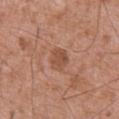notes: no biopsy performed (imaged during a skin exam); anatomic site: the abdomen; patient: male, roughly 75 years of age; diameter: ≈2.5 mm; acquisition: 15 mm crop, total-body photography.